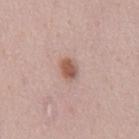This image is a 15 mm lesion crop taken from a total-body photograph. The total-body-photography lesion software estimated a shape eccentricity near 0.65 and a shape-asymmetry score of about 0.2 (0 = symmetric). The analysis additionally found a lesion color around L≈56 a*≈20 b*≈27 in CIELAB, roughly 12 lightness units darker than nearby skin, and a normalized border contrast of about 8.5. The analysis additionally found a border-irregularity index near 1.5/10 and radial color variation of about 1. It also reported a classifier nevus-likeness of about 95/100 and a detector confidence of about 100 out of 100 that the crop contains a lesion. Measured at roughly 3 mm in maximum diameter. The lesion is located on the abdomen. Imaged with white-light lighting. The patient is a male about 40 years old.